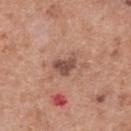This lesion was catalogued during total-body skin photography and was not selected for biopsy. A female subject, aged around 60. The recorded lesion diameter is about 3 mm. A roughly 15 mm field-of-view crop from a total-body skin photograph. The lesion is on the upper back.